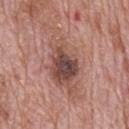This lesion was catalogued during total-body skin photography and was not selected for biopsy. On the back. Cropped from a total-body skin-imaging series; the visible field is about 15 mm. Automated image analysis of the tile measured an automated nevus-likeness rating near 80 out of 100 and a lesion-detection confidence of about 100/100. A male patient about 70 years old. Imaged with white-light lighting.